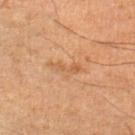Recorded during total-body skin imaging; not selected for excision or biopsy. The lesion is on the leg. Cropped from a whole-body photographic skin survey; the tile spans about 15 mm. The lesion's longest dimension is about 3.5 mm. A male patient approximately 65 years of age. An algorithmic analysis of the crop reported a mean CIELAB color near L≈47 a*≈19 b*≈32, about 6 CIELAB-L* units darker than the surrounding skin, and a normalized lesion–skin contrast near 5. This is a cross-polarized tile.